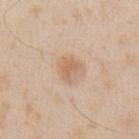Impression:
The lesion was tiled from a total-body skin photograph and was not biopsied.
Acquisition and patient details:
A male subject approximately 45 years of age. The lesion is on the left upper arm. A region of skin cropped from a whole-body photographic capture, roughly 15 mm wide.